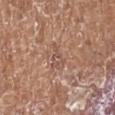The lesion was tiled from a total-body skin photograph and was not biopsied. Approximately 3.5 mm at its widest. From the right lower leg. Automated tile analysis of the lesion measured a classifier nevus-likeness of about 0/100 and lesion-presence confidence of about 85/100. A female patient, about 75 years old. Cropped from a total-body skin-imaging series; the visible field is about 15 mm. Captured under white-light illumination.